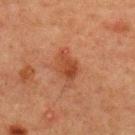No biopsy was performed on this lesion — it was imaged during a full skin examination and was not determined to be concerning. Automated tile analysis of the lesion measured a lesion area of about 7.5 mm², an eccentricity of roughly 0.8, and two-axis asymmetry of about 0.25. The software also gave internal color variation of about 3.5 on a 0–10 scale and a peripheral color-asymmetry measure near 1. The software also gave a classifier nevus-likeness of about 55/100 and lesion-presence confidence of about 100/100. This image is a 15 mm lesion crop taken from a total-body photograph. The patient is a male approximately 60 years of age. The lesion is located on the back.